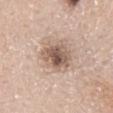notes = catalogued during a skin exam; not biopsied | location = the mid back | image = 15 mm crop, total-body photography | patient = male, aged around 40 | lesion diameter = ≈4 mm.